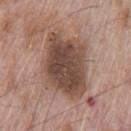Captured during whole-body skin photography for melanoma surveillance; the lesion was not biopsied. Measured at roughly 8 mm in maximum diameter. From the chest. A region of skin cropped from a whole-body photographic capture, roughly 15 mm wide. Automated image analysis of the tile measured a lesion area of about 33 mm², an eccentricity of roughly 0.75, and a symmetry-axis asymmetry near 0.25. And it measured an average lesion color of about L≈47 a*≈17 b*≈25 (CIELAB). And it measured a border-irregularity rating of about 3/10 and a within-lesion color-variation index near 5.5/10. The analysis additionally found a detector confidence of about 100 out of 100 that the crop contains a lesion. The tile uses white-light illumination. A male patient, aged 68–72.